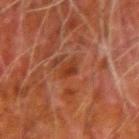The patient is a male approximately 80 years of age.
Captured under cross-polarized illumination.
A 15 mm close-up extracted from a 3D total-body photography capture.
The recorded lesion diameter is about 2.5 mm.
From the left upper arm.